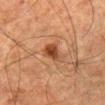Case summary:
• biopsy status: catalogued during a skin exam; not biopsied
• patient: male, about 80 years old
• automated lesion analysis: a mean CIELAB color near L≈35 a*≈21 b*≈30, roughly 11 lightness units darker than nearby skin, and a normalized border contrast of about 9.5
• location: the leg
• acquisition: total-body-photography crop, ~15 mm field of view
• tile lighting: cross-polarized
• size: about 2.5 mm